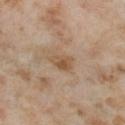workup: catalogued during a skin exam; not biopsied
location: the left lower leg
illumination: cross-polarized illumination
patient: female, roughly 55 years of age
imaging modality: ~15 mm crop, total-body skin-cancer survey
size: about 2.5 mm